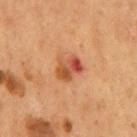The lesion was tiled from a total-body skin photograph and was not biopsied.
Cropped from a total-body skin-imaging series; the visible field is about 15 mm.
A male subject in their mid- to late 50s.
Longest diameter approximately 3 mm.
Imaged with cross-polarized lighting.
Automated tile analysis of the lesion measured an average lesion color of about L≈48 a*≈28 b*≈35 (CIELAB), about 11 CIELAB-L* units darker than the surrounding skin, and a lesion-to-skin contrast of about 8 (normalized; higher = more distinct). The software also gave a border-irregularity rating of about 3.5/10, a within-lesion color-variation index near 10/10, and radial color variation of about 4.5. The analysis additionally found a detector confidence of about 100 out of 100 that the crop contains a lesion.
From the mid back.